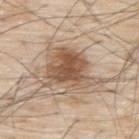Case summary:
- workup — catalogued during a skin exam; not biopsied
- illumination — white-light illumination
- size — ≈7 mm
- patient — male, roughly 80 years of age
- acquisition — ~15 mm tile from a whole-body skin photo
- anatomic site — the upper back